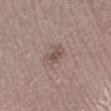diameter — ~2.5 mm (longest diameter) | body site — the right leg | imaging modality — total-body-photography crop, ~15 mm field of view | tile lighting — white-light | TBP lesion metrics — an area of roughly 4 mm² and an outline eccentricity of about 0.8 (0 = round, 1 = elongated); a border-irregularity index near 2.5/10 and a peripheral color-asymmetry measure near 1; an automated nevus-likeness rating near 10 out of 100 and lesion-presence confidence of about 100/100 | patient — male, roughly 70 years of age.